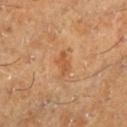body site — the leg | illumination — cross-polarized illumination | TBP lesion metrics — a lesion color around L≈51 a*≈23 b*≈38 in CIELAB, roughly 8 lightness units darker than nearby skin, and a lesion-to-skin contrast of about 6 (normalized; higher = more distinct); peripheral color asymmetry of about 0.5 | lesion diameter — about 3 mm | imaging modality — ~15 mm tile from a whole-body skin photo | patient — male, approximately 60 years of age.